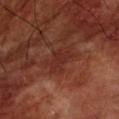- notes · no biopsy performed (imaged during a skin exam)
- acquisition · total-body-photography crop, ~15 mm field of view
- tile lighting · cross-polarized illumination
- site · the left forearm
- lesion size · ~2.5 mm (longest diameter)
- subject · male, aged approximately 70
- image-analysis metrics · a shape eccentricity near 0.7 and a shape-asymmetry score of about 0.5 (0 = symmetric); an automated nevus-likeness rating near 0 out of 100 and lesion-presence confidence of about 95/100